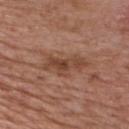A male patient, aged approximately 60. Automated image analysis of the tile measured an automated nevus-likeness rating near 0 out of 100. A 15 mm crop from a total-body photograph taken for skin-cancer surveillance. This is a white-light tile. About 5.5 mm across. The lesion is located on the chest.